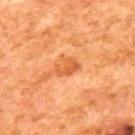Case summary:
* workup: catalogued during a skin exam; not biopsied
* subject: male, about 80 years old
* site: the upper back
* image source: ~15 mm tile from a whole-body skin photo